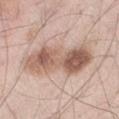Notes:
* biopsy status — no biopsy performed (imaged during a skin exam)
* size — about 8.5 mm
* image — ~15 mm tile from a whole-body skin photo
* patient — male, in their mid-40s
* site — the right thigh
* tile lighting — white-light